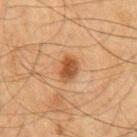follow-up: total-body-photography surveillance lesion; no biopsy | subject: male, aged 63–67 | lesion diameter: about 3 mm | automated lesion analysis: a lesion area of about 6 mm² and two-axis asymmetry of about 0.2; a lesion-to-skin contrast of about 8.5 (normalized; higher = more distinct); a border-irregularity index near 2/10, internal color variation of about 4 on a 0–10 scale, and a peripheral color-asymmetry measure near 1; a nevus-likeness score of about 95/100 and a detector confidence of about 100 out of 100 that the crop contains a lesion | tile lighting: cross-polarized illumination | imaging modality: ~15 mm tile from a whole-body skin photo | location: the chest.